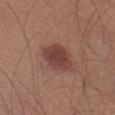workup: imaged on a skin check; not biopsied
illumination: white-light
patient: male, aged 48 to 52
acquisition: total-body-photography crop, ~15 mm field of view
diameter: ≈4.5 mm
location: the left thigh